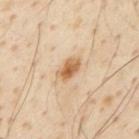Assessment:
The lesion was photographed on a routine skin check and not biopsied; there is no pathology result.
Acquisition and patient details:
This is a cross-polarized tile. The subject is a male aged 53 to 57. From the chest. This image is a 15 mm lesion crop taken from a total-body photograph. Measured at roughly 3 mm in maximum diameter.